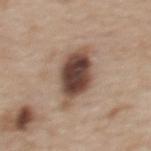{
  "biopsy_status": "not biopsied; imaged during a skin examination",
  "patient": {
    "sex": "female",
    "age_approx": 50
  },
  "lighting": "white-light",
  "image": {
    "source": "total-body photography crop",
    "field_of_view_mm": 15
  },
  "lesion_size": {
    "long_diameter_mm_approx": 5.0
  },
  "site": "upper back"
}